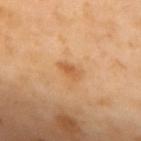notes — no biopsy performed (imaged during a skin exam) | automated lesion analysis — a footprint of about 3 mm², an outline eccentricity of about 0.85 (0 = round, 1 = elongated), and a symmetry-axis asymmetry near 0.25; border irregularity of about 2.5 on a 0–10 scale, a within-lesion color-variation index near 2/10, and peripheral color asymmetry of about 0.5; a classifier nevus-likeness of about 15/100 and a lesion-detection confidence of about 100/100 | subject — female, roughly 55 years of age | tile lighting — cross-polarized | anatomic site — the upper back | diameter — ~2.5 mm (longest diameter) | imaging modality — ~15 mm crop, total-body skin-cancer survey.